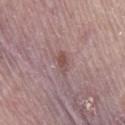- notes — catalogued during a skin exam; not biopsied
- image-analysis metrics — an area of roughly 3 mm², an eccentricity of roughly 0.85, and a symmetry-axis asymmetry near 0.2; border irregularity of about 2 on a 0–10 scale, a within-lesion color-variation index near 2/10, and radial color variation of about 0.5; a nevus-likeness score of about 0/100 and a detector confidence of about 100 out of 100 that the crop contains a lesion
- size — about 2.5 mm
- body site — the right thigh
- patient — female, aged 63–67
- image — ~15 mm tile from a whole-body skin photo
- tile lighting — white-light